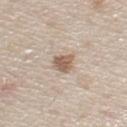Q: How was the tile lit?
A: white-light illumination
Q: What kind of image is this?
A: ~15 mm tile from a whole-body skin photo
Q: What is the anatomic site?
A: the chest
Q: Patient demographics?
A: male, approximately 60 years of age
Q: What is the lesion's diameter?
A: about 2.5 mm